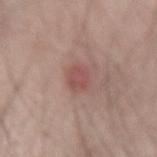No biopsy was performed on this lesion — it was imaged during a full skin examination and was not determined to be concerning. The tile uses white-light illumination. A roughly 15 mm field-of-view crop from a total-body skin photograph. On the arm. An algorithmic analysis of the crop reported a within-lesion color-variation index near 2.5/10 and peripheral color asymmetry of about 1. The patient is a male about 50 years old.